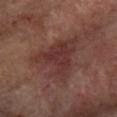Part of a total-body skin-imaging series; this lesion was reviewed on a skin check and was not flagged for biopsy.